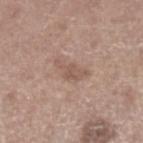Findings:
* notes · imaged on a skin check; not biopsied
* patient · male, aged around 65
* anatomic site · the left lower leg
* size · ~4 mm (longest diameter)
* image · 15 mm crop, total-body photography